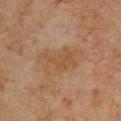Impression: The lesion was tiled from a total-body skin photograph and was not biopsied. Context: Located on the upper back. About 6 mm across. A roughly 15 mm field-of-view crop from a total-body skin photograph. A male patient aged 58 to 62.